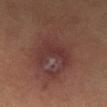Part of a total-body skin-imaging series; this lesion was reviewed on a skin check and was not flagged for biopsy. From the leg. A 15 mm close-up extracted from a 3D total-body photography capture. A male patient roughly 55 years of age. An algorithmic analysis of the crop reported a lesion area of about 12 mm² and a symmetry-axis asymmetry near 0.5. The software also gave a lesion color around L≈32 a*≈21 b*≈20 in CIELAB, roughly 6 lightness units darker than nearby skin, and a normalized lesion–skin contrast near 6. And it measured border irregularity of about 5.5 on a 0–10 scale, a color-variation rating of about 2.5/10, and radial color variation of about 1. And it measured a nevus-likeness score of about 0/100 and a detector confidence of about 85 out of 100 that the crop contains a lesion. Imaged with cross-polarized lighting. Longest diameter approximately 5 mm.